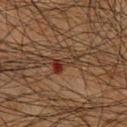workup: imaged on a skin check; not biopsied
illumination: cross-polarized illumination
body site: the chest
image: ~15 mm tile from a whole-body skin photo
patient: male, about 65 years old